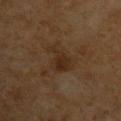biopsy_status: not biopsied; imaged during a skin examination
site: right upper arm
image:
  source: total-body photography crop
  field_of_view_mm: 15
patient:
  sex: male
  age_approx: 60